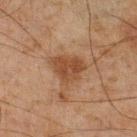Q: Is there a histopathology result?
A: total-body-photography surveillance lesion; no biopsy
Q: Who is the patient?
A: male, about 45 years old
Q: What is the anatomic site?
A: the right lower leg
Q: What is the imaging modality?
A: ~15 mm tile from a whole-body skin photo